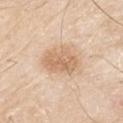Case summary:
* lesion size: about 4.5 mm
* patient: male, roughly 80 years of age
* body site: the right upper arm
* imaging modality: ~15 mm crop, total-body skin-cancer survey